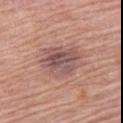<tbp_lesion>
  <biopsy_status>not biopsied; imaged during a skin examination</biopsy_status>
  <lesion_size>
    <long_diameter_mm_approx>5.0</long_diameter_mm_approx>
  </lesion_size>
  <site>left upper arm</site>
  <automated_metrics>
    <area_mm2_approx>15.0</area_mm2_approx>
    <eccentricity>0.6</eccentricity>
    <shape_asymmetry>0.2</shape_asymmetry>
    <cielab_L>52</cielab_L>
    <cielab_a>20</cielab_a>
    <cielab_b>21</cielab_b>
    <vs_skin_darker_L>10.0</vs_skin_darker_L>
    <vs_skin_contrast_norm>8.0</vs_skin_contrast_norm>
  </automated_metrics>
  <image>
    <source>total-body photography crop</source>
    <field_of_view_mm>15</field_of_view_mm>
  </image>
  <patient>
    <sex>male</sex>
    <age_approx>80</age_approx>
  </patient>
</tbp_lesion>